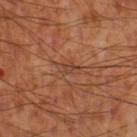Q: Is there a histopathology result?
A: imaged on a skin check; not biopsied
Q: Lesion location?
A: the leg
Q: Automated lesion metrics?
A: a lesion color around L≈43 a*≈23 b*≈32 in CIELAB; a classifier nevus-likeness of about 0/100 and lesion-presence confidence of about 70/100
Q: What is the lesion's diameter?
A: ~2.5 mm (longest diameter)
Q: How was the tile lit?
A: cross-polarized
Q: What kind of image is this?
A: ~15 mm crop, total-body skin-cancer survey
Q: Who is the patient?
A: male, approximately 55 years of age